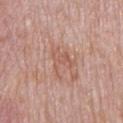Imaged during a routine full-body skin examination; the lesion was not biopsied and no histopathology is available. A male subject aged 73–77. From the mid back. This image is a 15 mm lesion crop taken from a total-body photograph. Captured under white-light illumination.